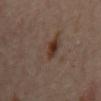| field | value |
|---|---|
| workup | no biopsy performed (imaged during a skin exam) |
| image source | ~15 mm crop, total-body skin-cancer survey |
| patient | female, about 60 years old |
| lighting | cross-polarized |
| body site | the mid back |